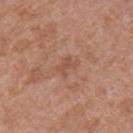biopsy_status: not biopsied; imaged during a skin examination
patient:
  sex: female
  age_approx: 40
image:
  source: total-body photography crop
  field_of_view_mm: 15
site: arm